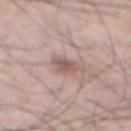Impression: No biopsy was performed on this lesion — it was imaged during a full skin examination and was not determined to be concerning. Clinical summary: A 15 mm crop from a total-body photograph taken for skin-cancer surveillance. A male subject aged 53–57. Located on the mid back.